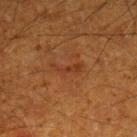<record>
  <biopsy_status>not biopsied; imaged during a skin examination</biopsy_status>
  <site>leg</site>
  <lighting>cross-polarized</lighting>
  <lesion_size>
    <long_diameter_mm_approx>3.5</long_diameter_mm_approx>
  </lesion_size>
  <image>
    <source>total-body photography crop</source>
    <field_of_view_mm>15</field_of_view_mm>
  </image>
  <patient>
    <sex>male</sex>
    <age_approx>60</age_approx>
  </patient>
</record>